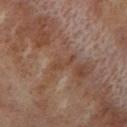Case summary:
• biopsy status · imaged on a skin check; not biopsied
• site · the left lower leg
• imaging modality · 15 mm crop, total-body photography
• tile lighting · cross-polarized illumination
• subject · female, aged 48 to 52
• automated metrics · border irregularity of about 5 on a 0–10 scale, a within-lesion color-variation index near 1/10, and a peripheral color-asymmetry measure near 0
• lesion size · about 3.5 mm